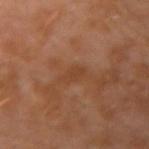<case>
  <biopsy_status>not biopsied; imaged during a skin examination</biopsy_status>
  <lighting>cross-polarized</lighting>
  <patient>
    <sex>male</sex>
    <age_approx>30</age_approx>
  </patient>
  <site>left arm</site>
  <automated_metrics>
    <area_mm2_approx>3.5</area_mm2_approx>
    <eccentricity>0.9</eccentricity>
    <border_irregularity_0_10>4.0</border_irregularity_0_10>
    <color_variation_0_10>0.5</color_variation_0_10>
    <peripheral_color_asymmetry>0.0</peripheral_color_asymmetry>
  </automated_metrics>
  <lesion_size>
    <long_diameter_mm_approx>3.0</long_diameter_mm_approx>
  </lesion_size>
  <image>
    <source>total-body photography crop</source>
    <field_of_view_mm>15</field_of_view_mm>
  </image>
</case>